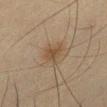Clinical summary:
Cropped from a whole-body photographic skin survey; the tile spans about 15 mm. The lesion is located on the left thigh. Approximately 3.5 mm at its widest. The total-body-photography lesion software estimated a lesion area of about 7.5 mm², a shape eccentricity near 0.5, and two-axis asymmetry of about 0.3. The analysis additionally found a lesion color around L≈40 a*≈12 b*≈26 in CIELAB, roughly 6 lightness units darker than nearby skin, and a normalized border contrast of about 6. The software also gave a border-irregularity index near 3/10, a within-lesion color-variation index near 2.5/10, and a peripheral color-asymmetry measure near 1. Captured under cross-polarized illumination. A male subject, aged around 65.